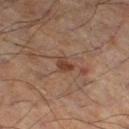Q: Was a biopsy performed?
A: total-body-photography surveillance lesion; no biopsy
Q: Lesion location?
A: the leg
Q: Who is the patient?
A: male, aged around 55
Q: What is the imaging modality?
A: ~15 mm tile from a whole-body skin photo